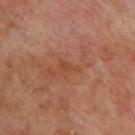A male subject in their 70s. Located on the upper back. Cropped from a whole-body photographic skin survey; the tile spans about 15 mm.